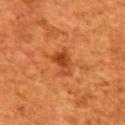Impression:
Captured during whole-body skin photography for melanoma surveillance; the lesion was not biopsied.
Context:
A male patient, aged approximately 45. From the upper back. A 15 mm close-up tile from a total-body photography series done for melanoma screening.